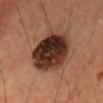An algorithmic analysis of the crop reported a mean CIELAB color near L≈28 a*≈19 b*≈23, about 18 CIELAB-L* units darker than the surrounding skin, and a normalized border contrast of about 15.5. Longest diameter approximately 6.5 mm. This image is a 15 mm lesion crop taken from a total-body photograph. Located on the head or neck. A male patient in their mid-30s. Captured under cross-polarized illumination.